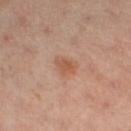Imaged during a routine full-body skin examination; the lesion was not biopsied and no histopathology is available.
A close-up tile cropped from a whole-body skin photograph, about 15 mm across.
A female patient aged around 40.
This is a cross-polarized tile.
On the right leg.
Approximately 2.5 mm at its widest.